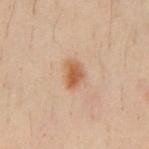The lesion was tiled from a total-body skin photograph and was not biopsied.
The lesion is located on the chest.
Longest diameter approximately 3 mm.
Cropped from a total-body skin-imaging series; the visible field is about 15 mm.
The subject is a male aged around 30.
Captured under cross-polarized illumination.